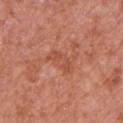Q: Is there a histopathology result?
A: catalogued during a skin exam; not biopsied
Q: Lesion location?
A: the left upper arm
Q: How was the tile lit?
A: white-light
Q: Patient demographics?
A: male, aged 63–67
Q: How large is the lesion?
A: about 3.5 mm
Q: What kind of image is this?
A: ~15 mm tile from a whole-body skin photo
Q: What did automated image analysis measure?
A: a border-irregularity index near 3/10, a color-variation rating of about 2/10, and a peripheral color-asymmetry measure near 0.5